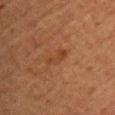Background:
The lesion is located on the chest. An algorithmic analysis of the crop reported border irregularity of about 4 on a 0–10 scale, internal color variation of about 0.5 on a 0–10 scale, and radial color variation of about 0. The analysis additionally found an automated nevus-likeness rating near 0 out of 100. A 15 mm crop from a total-body photograph taken for skin-cancer surveillance. A female subject, approximately 70 years of age. The recorded lesion diameter is about 3 mm. Imaged with cross-polarized lighting.